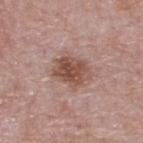Part of a total-body skin-imaging series; this lesion was reviewed on a skin check and was not flagged for biopsy. The total-body-photography lesion software estimated a lesion area of about 12 mm², an outline eccentricity of about 0.7 (0 = round, 1 = elongated), and a symmetry-axis asymmetry near 0.2. On the upper back. This is a white-light tile. The recorded lesion diameter is about 4.5 mm. The subject is a male roughly 55 years of age. A region of skin cropped from a whole-body photographic capture, roughly 15 mm wide.